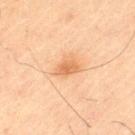| key | value |
|---|---|
| follow-up | total-body-photography surveillance lesion; no biopsy |
| image source | ~15 mm tile from a whole-body skin photo |
| TBP lesion metrics | border irregularity of about 3 on a 0–10 scale, a color-variation rating of about 2/10, and peripheral color asymmetry of about 0.5 |
| size | about 3 mm |
| site | the left thigh |
| patient | male, aged 63–67 |